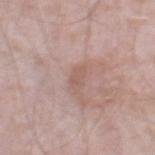Notes:
– follow-up: catalogued during a skin exam; not biopsied
– diameter: ≈2.5 mm
– image source: ~15 mm crop, total-body skin-cancer survey
– patient: male, roughly 50 years of age
– lighting: white-light
– location: the leg
– image-analysis metrics: a border-irregularity index near 2.5/10, a within-lesion color-variation index near 1/10, and peripheral color asymmetry of about 0.5; a nevus-likeness score of about 0/100 and a detector confidence of about 100 out of 100 that the crop contains a lesion